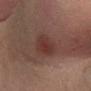{"biopsy_status": "not biopsied; imaged during a skin examination", "lighting": "cross-polarized", "image": {"source": "total-body photography crop", "field_of_view_mm": 15}, "patient": {"sex": "male", "age_approx": 65}, "site": "leg", "automated_metrics": {"eccentricity": 0.65, "shape_asymmetry": 0.25, "cielab_L": 29, "cielab_a": 17, "cielab_b": 19, "vs_skin_darker_L": 7.0, "vs_skin_contrast_norm": 7.0, "border_irregularity_0_10": 2.5, "peripheral_color_asymmetry": 1.0}}